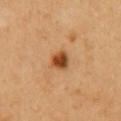Q: Was a biopsy performed?
A: imaged on a skin check; not biopsied
Q: Patient demographics?
A: male, in their 50s
Q: What kind of image is this?
A: ~15 mm tile from a whole-body skin photo
Q: Lesion location?
A: the chest